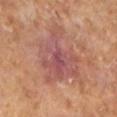Part of a total-body skin-imaging series; this lesion was reviewed on a skin check and was not flagged for biopsy.
The subject is a male aged around 65.
The lesion's longest dimension is about 7.5 mm.
A close-up tile cropped from a whole-body skin photograph, about 15 mm across.
The tile uses cross-polarized illumination.
The total-body-photography lesion software estimated a lesion color around L≈51 a*≈24 b*≈24 in CIELAB. It also reported an automated nevus-likeness rating near 0 out of 100.